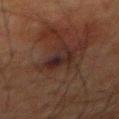notes — total-body-photography surveillance lesion; no biopsy | lighting — cross-polarized illumination | subject — male, aged approximately 80 | anatomic site — the abdomen | diameter — ≈4 mm | image — 15 mm crop, total-body photography.